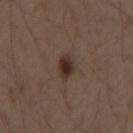{"biopsy_status": "not biopsied; imaged during a skin examination", "patient": {"sex": "male", "age_approx": 50}, "image": {"source": "total-body photography crop", "field_of_view_mm": 15}, "lighting": "white-light", "lesion_size": {"long_diameter_mm_approx": 3.0}, "site": "left upper arm"}